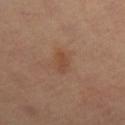Clinical impression:
Recorded during total-body skin imaging; not selected for excision or biopsy.
Context:
A 15 mm crop from a total-body photograph taken for skin-cancer surveillance. A female patient about 70 years old. Longest diameter approximately 2.5 mm. Located on the leg. The total-body-photography lesion software estimated an average lesion color of about L≈47 a*≈20 b*≈31 (CIELAB), a lesion–skin lightness drop of about 6, and a lesion-to-skin contrast of about 5.5 (normalized; higher = more distinct). It also reported a border-irregularity rating of about 1.5/10 and peripheral color asymmetry of about 1. The software also gave a nevus-likeness score of about 25/100 and a lesion-detection confidence of about 100/100.